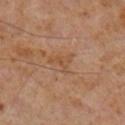follow-up: no biopsy performed (imaged during a skin exam) | illumination: cross-polarized illumination | TBP lesion metrics: a footprint of about 4 mm²; a lesion color around L≈46 a*≈19 b*≈31 in CIELAB, roughly 5 lightness units darker than nearby skin, and a normalized border contrast of about 5; a lesion-detection confidence of about 100/100 | diameter: ≈3 mm | site: the right lower leg | acquisition: ~15 mm crop, total-body skin-cancer survey | subject: male, aged 58 to 62.